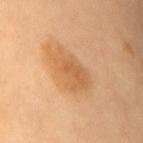follow-up = no biopsy performed (imaged during a skin exam)
imaging modality = ~15 mm tile from a whole-body skin photo
site = the mid back
image-analysis metrics = a lesion color around L≈59 a*≈21 b*≈40 in CIELAB and a normalized border contrast of about 6; a border-irregularity rating of about 3.5/10, a color-variation rating of about 3.5/10, and radial color variation of about 1; an automated nevus-likeness rating near 65 out of 100 and a detector confidence of about 100 out of 100 that the crop contains a lesion
patient = female, aged approximately 70